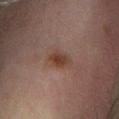No biopsy was performed on this lesion — it was imaged during a full skin examination and was not determined to be concerning. A male subject, aged approximately 65. The lesion is located on the right lower leg. The lesion-visualizer software estimated a footprint of about 5.5 mm², an outline eccentricity of about 0.75 (0 = round, 1 = elongated), and a symmetry-axis asymmetry near 0.25. A region of skin cropped from a whole-body photographic capture, roughly 15 mm wide. Longest diameter approximately 3 mm.